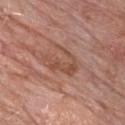This lesion was catalogued during total-body skin photography and was not selected for biopsy.